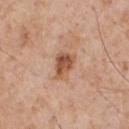  biopsy_status: not biopsied; imaged during a skin examination
  automated_metrics:
    vs_skin_darker_L: 12.0
    vs_skin_contrast_norm: 8.0
    border_irregularity_0_10: 2.5
    color_variation_0_10: 5.5
    peripheral_color_asymmetry: 2.0
  lighting: white-light
  site: chest
  image:
    source: total-body photography crop
    field_of_view_mm: 15
  lesion_size:
    long_diameter_mm_approx: 3.5
  patient:
    sex: male
    age_approx: 60